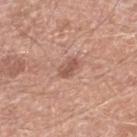Captured during whole-body skin photography for melanoma surveillance; the lesion was not biopsied. A 15 mm close-up extracted from a 3D total-body photography capture. The lesion is on the leg. Approximately 2.5 mm at its widest. A male patient, about 80 years old. The tile uses white-light illumination. The lesion-visualizer software estimated an area of roughly 4 mm², an outline eccentricity of about 0.8 (0 = round, 1 = elongated), and a shape-asymmetry score of about 0.2 (0 = symmetric). The software also gave a classifier nevus-likeness of about 45/100.